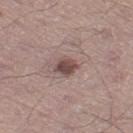anatomic site: the left thigh; patient: male, in their 60s; acquisition: ~15 mm tile from a whole-body skin photo; size: ≈3 mm.